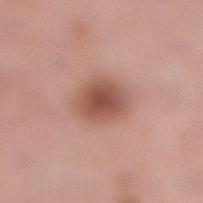biopsy status: catalogued during a skin exam; not biopsied
subject: female, about 40 years old
anatomic site: the right lower leg
image source: ~15 mm crop, total-body skin-cancer survey